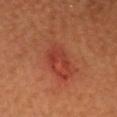biopsy_status: not biopsied; imaged during a skin examination
lesion_size:
  long_diameter_mm_approx: 3.0
image:
  source: total-body photography crop
  field_of_view_mm: 15
patient:
  sex: male
  age_approx: 65
site: head or neck